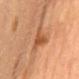biopsy_status: not biopsied; imaged during a skin examination
lighting: cross-polarized
image:
  source: total-body photography crop
  field_of_view_mm: 15
lesion_size:
  long_diameter_mm_approx: 5.0
patient:
  sex: female
  age_approx: 60
automated_metrics:
  cielab_L: 47
  cielab_a: 23
  cielab_b: 34
  vs_skin_darker_L: 10.0
  vs_skin_contrast_norm: 7.5
  nevus_likeness_0_100: 0
  lesion_detection_confidence_0_100: 95
site: mid back